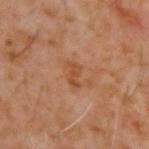Approximately 3 mm at its widest.
Imaged with cross-polarized lighting.
Cropped from a total-body skin-imaging series; the visible field is about 15 mm.
The subject is a male about 60 years old.
The total-body-photography lesion software estimated an area of roughly 3 mm² and a shape-asymmetry score of about 0.4 (0 = symmetric). The analysis additionally found a lesion color around L≈45 a*≈23 b*≈34 in CIELAB, about 7 CIELAB-L* units darker than the surrounding skin, and a normalized border contrast of about 6.5. And it measured a border-irregularity rating of about 4.5/10.
The lesion is on the back.